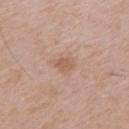The lesion was photographed on a routine skin check and not biopsied; there is no pathology result.